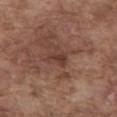<record>
<biopsy_status>not biopsied; imaged during a skin examination</biopsy_status>
<lighting>white-light</lighting>
<patient>
  <sex>male</sex>
  <age_approx>75</age_approx>
</patient>
<automated_metrics>
  <area_mm2_approx>3.5</area_mm2_approx>
  <eccentricity>0.8</eccentricity>
  <cielab_L>38</cielab_L>
  <cielab_a>20</cielab_a>
  <cielab_b>24</cielab_b>
  <vs_skin_contrast_norm>6.5</vs_skin_contrast_norm>
  <nevus_likeness_0_100>0</nevus_likeness_0_100>
  <lesion_detection_confidence_0_100>90</lesion_detection_confidence_0_100>
</automated_metrics>
<image>
  <source>total-body photography crop</source>
  <field_of_view_mm>15</field_of_view_mm>
</image>
<lesion_size>
  <long_diameter_mm_approx>2.5</long_diameter_mm_approx>
</lesion_size>
<site>abdomen</site>
</record>